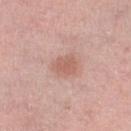Part of a total-body skin-imaging series; this lesion was reviewed on a skin check and was not flagged for biopsy.
From the right lower leg.
Cropped from a total-body skin-imaging series; the visible field is about 15 mm.
Imaged with white-light lighting.
The lesion-visualizer software estimated an area of roughly 5 mm² and two-axis asymmetry of about 0.2. The software also gave a lesion color around L≈60 a*≈23 b*≈26 in CIELAB, roughly 9 lightness units darker than nearby skin, and a normalized border contrast of about 6. The software also gave border irregularity of about 2 on a 0–10 scale, a within-lesion color-variation index near 2/10, and peripheral color asymmetry of about 0.5. And it measured an automated nevus-likeness rating near 60 out of 100 and a lesion-detection confidence of about 100/100.
A male subject, approximately 60 years of age.
Measured at roughly 3 mm in maximum diameter.